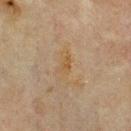The recorded lesion diameter is about 2.5 mm. Captured under cross-polarized illumination. A male patient aged approximately 70. On the chest. The lesion-visualizer software estimated an area of roughly 2.5 mm², an outline eccentricity of about 0.8 (0 = round, 1 = elongated), and a symmetry-axis asymmetry near 0.5. The analysis additionally found roughly 5 lightness units darker than nearby skin. A 15 mm close-up tile from a total-body photography series done for melanoma screening.